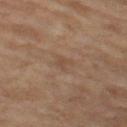No biopsy was performed on this lesion — it was imaged during a full skin examination and was not determined to be concerning. Longest diameter approximately 2.5 mm. A female patient aged 68–72. An algorithmic analysis of the crop reported a footprint of about 2.5 mm², a shape eccentricity near 0.8, and two-axis asymmetry of about 0.4. Cropped from a whole-body photographic skin survey; the tile spans about 15 mm. Located on the left thigh.